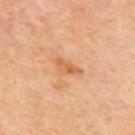Recorded during total-body skin imaging; not selected for excision or biopsy.
A region of skin cropped from a whole-body photographic capture, roughly 15 mm wide.
A male subject aged approximately 70.
The tile uses cross-polarized illumination.
An algorithmic analysis of the crop reported a nevus-likeness score of about 0/100 and lesion-presence confidence of about 100/100.
Approximately 3 mm at its widest.
The lesion is on the chest.